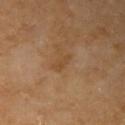Assessment:
Imaged during a routine full-body skin examination; the lesion was not biopsied and no histopathology is available.
Background:
An algorithmic analysis of the crop reported a lesion–skin lightness drop of about 6 and a normalized lesion–skin contrast near 5. About 3 mm across. From the right upper arm. A female patient approximately 70 years of age. Cropped from a whole-body photographic skin survey; the tile spans about 15 mm.